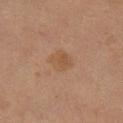This lesion was catalogued during total-body skin photography and was not selected for biopsy. The lesion is on the leg. The lesion's longest dimension is about 3 mm. A region of skin cropped from a whole-body photographic capture, roughly 15 mm wide. Automated tile analysis of the lesion measured a lesion color around L≈43 a*≈16 b*≈28 in CIELAB. It also reported a border-irregularity rating of about 1.5/10, a within-lesion color-variation index near 2/10, and radial color variation of about 0.5. And it measured a classifier nevus-likeness of about 10/100. The tile uses cross-polarized illumination. A female patient aged 38–42.